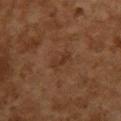Cropped from a total-body skin-imaging series; the visible field is about 15 mm.
About 3 mm across.
The lesion is on the upper back.
Captured under cross-polarized illumination.
An algorithmic analysis of the crop reported a lesion area of about 3.5 mm², a shape eccentricity near 0.9, and a symmetry-axis asymmetry near 0.35. It also reported a lesion–skin lightness drop of about 5 and a lesion-to-skin contrast of about 5.5 (normalized; higher = more distinct). The analysis additionally found a classifier nevus-likeness of about 0/100 and lesion-presence confidence of about 100/100.
A female patient aged 58 to 62.